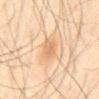Captured during whole-body skin photography for melanoma surveillance; the lesion was not biopsied.